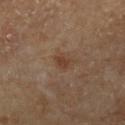The lesion was tiled from a total-body skin photograph and was not biopsied. The lesion is located on the right forearm. Longest diameter approximately 2 mm. A male patient, in their mid- to late 50s. A close-up tile cropped from a whole-body skin photograph, about 15 mm across.